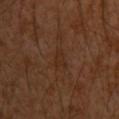Part of a total-body skin-imaging series; this lesion was reviewed on a skin check and was not flagged for biopsy.
Located on the chest.
The subject is a male aged 28–32.
A 15 mm close-up tile from a total-body photography series done for melanoma screening.